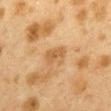• workup: no biopsy performed (imaged during a skin exam)
• diameter: ≈3 mm
• image source: ~15 mm crop, total-body skin-cancer survey
• lighting: cross-polarized
• subject: female, aged approximately 40
• anatomic site: the mid back